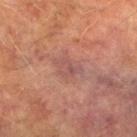| field | value |
|---|---|
| workup | no biopsy performed (imaged during a skin exam) |
| patient | male, roughly 75 years of age |
| image source | 15 mm crop, total-body photography |
| anatomic site | the leg |